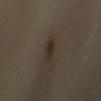lesion size = ≈3 mm | image = ~15 mm tile from a whole-body skin photo | location = the right upper arm | subject = female, about 50 years old | illumination = cross-polarized | histopathology = an atypical melanocytic neoplasm (borderline).